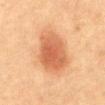Captured during whole-body skin photography for melanoma surveillance; the lesion was not biopsied.
Imaged with cross-polarized lighting.
On the abdomen.
The subject is a female aged 58–62.
A 15 mm close-up tile from a total-body photography series done for melanoma screening.